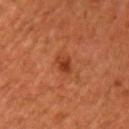Q: Was this lesion biopsied?
A: no biopsy performed (imaged during a skin exam)
Q: Where on the body is the lesion?
A: the arm
Q: Who is the patient?
A: male, aged around 45
Q: What did automated image analysis measure?
A: internal color variation of about 3 on a 0–10 scale and radial color variation of about 1
Q: How large is the lesion?
A: ≈3 mm
Q: What lighting was used for the tile?
A: cross-polarized illumination
Q: How was this image acquired?
A: ~15 mm tile from a whole-body skin photo